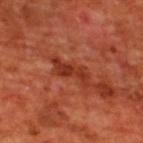<case>
  <biopsy_status>not biopsied; imaged during a skin examination</biopsy_status>
  <automated_metrics>
    <border_irregularity_0_10>6.0</border_irregularity_0_10>
    <color_variation_0_10>3.0</color_variation_0_10>
    <peripheral_color_asymmetry>1.0</peripheral_color_asymmetry>
  </automated_metrics>
  <lesion_size>
    <long_diameter_mm_approx>5.0</long_diameter_mm_approx>
  </lesion_size>
  <lighting>cross-polarized</lighting>
  <patient>
    <sex>male</sex>
    <age_approx>70</age_approx>
  </patient>
  <image>
    <source>total-body photography crop</source>
    <field_of_view_mm>15</field_of_view_mm>
  </image>
  <site>upper back</site>
</case>